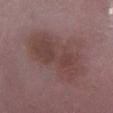Q: Illumination type?
A: white-light illumination
Q: Who is the patient?
A: male, aged 48 to 52
Q: What is the lesion's diameter?
A: ~9.5 mm (longest diameter)
Q: How was this image acquired?
A: 15 mm crop, total-body photography
Q: Lesion location?
A: the right lower leg